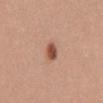Part of a total-body skin-imaging series; this lesion was reviewed on a skin check and was not flagged for biopsy.
The lesion is on the mid back.
About 2.5 mm across.
A female subject about 35 years old.
Imaged with white-light lighting.
A lesion tile, about 15 mm wide, cut from a 3D total-body photograph.
An algorithmic analysis of the crop reported a border-irregularity index near 1.5/10, a color-variation rating of about 3/10, and radial color variation of about 1.